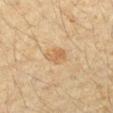Impression: Part of a total-body skin-imaging series; this lesion was reviewed on a skin check and was not flagged for biopsy. Clinical summary: Captured under cross-polarized illumination. Longest diameter approximately 2.5 mm. Located on the right forearm. A female patient aged 38–42. A region of skin cropped from a whole-body photographic capture, roughly 15 mm wide.